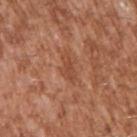Clinical summary: A male patient in their mid-40s. Approximately 3 mm at its widest. The lesion is on the right upper arm. Cropped from a whole-body photographic skin survey; the tile spans about 15 mm. Captured under white-light illumination.